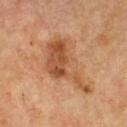Captured under cross-polarized illumination. The recorded lesion diameter is about 8 mm. The lesion is on the chest. A male patient, roughly 65 years of age. An algorithmic analysis of the crop reported a shape eccentricity near 0.85 and two-axis asymmetry of about 0.6. And it measured roughly 11 lightness units darker than nearby skin and a lesion-to-skin contrast of about 8 (normalized; higher = more distinct). The analysis additionally found a within-lesion color-variation index near 5/10 and a peripheral color-asymmetry measure near 2. A 15 mm close-up tile from a total-body photography series done for melanoma screening.